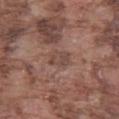Captured during whole-body skin photography for melanoma surveillance; the lesion was not biopsied.